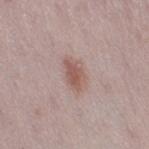Clinical impression: Part of a total-body skin-imaging series; this lesion was reviewed on a skin check and was not flagged for biopsy. Context: A region of skin cropped from a whole-body photographic capture, roughly 15 mm wide. The patient is a female aged 28 to 32. The lesion is on the right thigh.